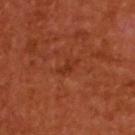An algorithmic analysis of the crop reported an area of roughly 3 mm² and a symmetry-axis asymmetry near 0.4. The software also gave a border-irregularity index near 4.5/10 and a color-variation rating of about 0.5/10. The patient is a female approximately 55 years of age. This image is a 15 mm lesion crop taken from a total-body photograph. Imaged with cross-polarized lighting. The lesion is on the upper back.